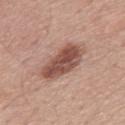A male subject aged 63 to 67. A 15 mm close-up tile from a total-body photography series done for melanoma screening. Located on the back. Imaged with white-light lighting. The total-body-photography lesion software estimated an outline eccentricity of about 0.9 (0 = round, 1 = elongated) and a symmetry-axis asymmetry near 0.2. It also reported a border-irregularity index near 2.5/10 and internal color variation of about 4.5 on a 0–10 scale. The software also gave an automated nevus-likeness rating near 65 out of 100 and lesion-presence confidence of about 100/100. About 6 mm across.